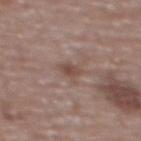notes: catalogued during a skin exam; not biopsied | diameter: ≈3 mm | location: the back | acquisition: 15 mm crop, total-body photography | image-analysis metrics: an area of roughly 3.5 mm² and a shape-asymmetry score of about 0.25 (0 = symmetric); an average lesion color of about L≈48 a*≈17 b*≈22 (CIELAB), roughly 8 lightness units darker than nearby skin, and a normalized border contrast of about 6.5 | patient: female, aged 63–67.